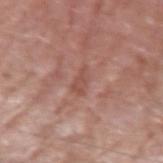This lesion was catalogued during total-body skin photography and was not selected for biopsy.
A close-up tile cropped from a whole-body skin photograph, about 15 mm across.
From the left upper arm.
The subject is a male approximately 80 years of age.